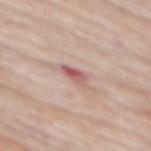Imaged during a routine full-body skin examination; the lesion was not biopsied and no histopathology is available.
A 15 mm close-up extracted from a 3D total-body photography capture.
On the mid back.
This is a white-light tile.
The lesion's longest dimension is about 3 mm.
The subject is a male aged 83 or older.